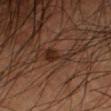Captured during whole-body skin photography for melanoma surveillance; the lesion was not biopsied.
From the left forearm.
Measured at roughly 4 mm in maximum diameter.
Cropped from a whole-body photographic skin survey; the tile spans about 15 mm.
A male subject, roughly 55 years of age.
Automated image analysis of the tile measured an area of roughly 5 mm² and two-axis asymmetry of about 0.55. And it measured a mean CIELAB color near L≈25 a*≈17 b*≈23, roughly 7 lightness units darker than nearby skin, and a lesion-to-skin contrast of about 8 (normalized; higher = more distinct).